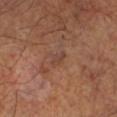Impression:
Recorded during total-body skin imaging; not selected for excision or biopsy.
Context:
From the left lower leg. Captured under cross-polarized illumination. Automated tile analysis of the lesion measured a footprint of about 2.5 mm², an outline eccentricity of about 0.85 (0 = round, 1 = elongated), and two-axis asymmetry of about 0.25. The analysis additionally found an automated nevus-likeness rating near 0 out of 100 and lesion-presence confidence of about 100/100. Approximately 2.5 mm at its widest. A male subject, about 65 years old. A roughly 15 mm field-of-view crop from a total-body skin photograph.